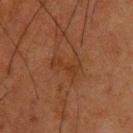Captured during whole-body skin photography for melanoma surveillance; the lesion was not biopsied. A male patient, roughly 35 years of age. This is a cross-polarized tile. From the upper back. A region of skin cropped from a whole-body photographic capture, roughly 15 mm wide. Automated image analysis of the tile measured an average lesion color of about L≈27 a*≈18 b*≈27 (CIELAB) and a normalized border contrast of about 5.5. The analysis additionally found a nevus-likeness score of about 0/100 and a detector confidence of about 100 out of 100 that the crop contains a lesion. Longest diameter approximately 3.5 mm.